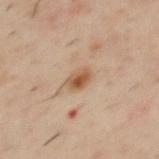Q: What are the patient's age and sex?
A: male, roughly 40 years of age
Q: Lesion location?
A: the upper back
Q: What is the imaging modality?
A: ~15 mm tile from a whole-body skin photo
Q: Lesion size?
A: ≈2.5 mm